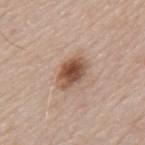Captured during whole-body skin photography for melanoma surveillance; the lesion was not biopsied. An algorithmic analysis of the crop reported a lesion color around L≈52 a*≈19 b*≈29 in CIELAB, roughly 14 lightness units darker than nearby skin, and a normalized lesion–skin contrast near 10. Captured under white-light illumination. From the mid back. Longest diameter approximately 4 mm. A male patient, aged approximately 65. A 15 mm close-up tile from a total-body photography series done for melanoma screening.